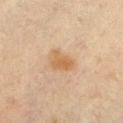notes = total-body-photography surveillance lesion; no biopsy
tile lighting = cross-polarized
patient = female, aged approximately 50
acquisition = ~15 mm tile from a whole-body skin photo
location = the chest